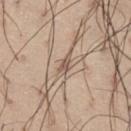biopsy_status: not biopsied; imaged during a skin examination
image:
  source: total-body photography crop
  field_of_view_mm: 15
site: leg
lesion_size:
  long_diameter_mm_approx: 3.0
lighting: white-light
automated_metrics:
  area_mm2_approx: 3.5
  eccentricity: 0.9
  shape_asymmetry: 0.4
  border_irregularity_0_10: 4.5
patient:
  sex: male
  age_approx: 55